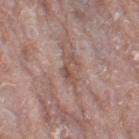This lesion was catalogued during total-body skin photography and was not selected for biopsy.
The tile uses white-light illumination.
Cropped from a total-body skin-imaging series; the visible field is about 15 mm.
The recorded lesion diameter is about 3.5 mm.
On the leg.
The patient is a female in their mid-70s.